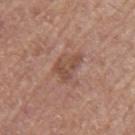Findings:
* biopsy status — total-body-photography surveillance lesion; no biopsy
* lesion size — ~3.5 mm (longest diameter)
* lighting — white-light illumination
* body site — the left upper arm
* automated metrics — a lesion area of about 6.5 mm², an eccentricity of roughly 0.65, and two-axis asymmetry of about 0.5; a border-irregularity rating of about 5/10 and radial color variation of about 0.5; an automated nevus-likeness rating near 0 out of 100
* imaging modality — ~15 mm tile from a whole-body skin photo
* subject — male, approximately 65 years of age